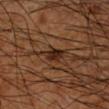Imaged during a routine full-body skin examination; the lesion was not biopsied and no histopathology is available.
A male subject aged 63 to 67.
The lesion is located on the right forearm.
The lesion-visualizer software estimated an area of roughly 4 mm².
Cropped from a whole-body photographic skin survey; the tile spans about 15 mm.
Measured at roughly 2.5 mm in maximum diameter.